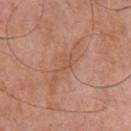Notes:
- notes — total-body-photography surveillance lesion; no biopsy
- acquisition — total-body-photography crop, ~15 mm field of view
- illumination — white-light
- automated lesion analysis — a nevus-likeness score of about 0/100
- patient — male, about 70 years old
- location — the chest